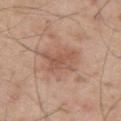notes: imaged on a skin check; not biopsied | automated lesion analysis: a lesion color around L≈54 a*≈22 b*≈29 in CIELAB, about 9 CIELAB-L* units darker than the surrounding skin, and a lesion-to-skin contrast of about 6 (normalized; higher = more distinct); a classifier nevus-likeness of about 20/100 | illumination: white-light | acquisition: ~15 mm tile from a whole-body skin photo | patient: male, about 55 years old | diameter: ≈4.5 mm | location: the upper back.